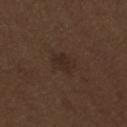{"biopsy_status": "not biopsied; imaged during a skin examination", "lesion_size": {"long_diameter_mm_approx": 3.0}, "patient": {"sex": "male", "age_approx": 70}, "lighting": "white-light", "image": {"source": "total-body photography crop", "field_of_view_mm": 15}, "site": "mid back", "automated_metrics": {"cielab_L": 26, "cielab_a": 14, "cielab_b": 21, "vs_skin_darker_L": 5.0}}